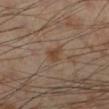From the left lower leg. A roughly 15 mm field-of-view crop from a total-body skin photograph. Automated tile analysis of the lesion measured a lesion area of about 3.5 mm². The software also gave a border-irregularity rating of about 3.5/10, internal color variation of about 3.5 on a 0–10 scale, and a peripheral color-asymmetry measure near 1. Imaged with cross-polarized lighting. The patient is a male in their mid- to late 50s.